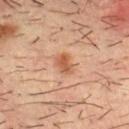{
  "biopsy_status": "not biopsied; imaged during a skin examination",
  "image": {
    "source": "total-body photography crop",
    "field_of_view_mm": 15
  },
  "lighting": "cross-polarized",
  "patient": {
    "sex": "male",
    "age_approx": 35
  },
  "site": "chest"
}